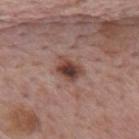Captured during whole-body skin photography for melanoma surveillance; the lesion was not biopsied.